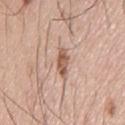Q: Was a biopsy performed?
A: no biopsy performed (imaged during a skin exam)
Q: Patient demographics?
A: male, approximately 75 years of age
Q: Lesion size?
A: ~4 mm (longest diameter)
Q: What is the imaging modality?
A: ~15 mm crop, total-body skin-cancer survey
Q: What is the anatomic site?
A: the chest
Q: What lighting was used for the tile?
A: white-light